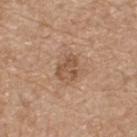A male subject, approximately 70 years of age. The lesion-visualizer software estimated a footprint of about 6.5 mm², a shape eccentricity near 0.55, and a symmetry-axis asymmetry near 0.3. And it measured an average lesion color of about L≈53 a*≈19 b*≈31 (CIELAB), about 9 CIELAB-L* units darker than the surrounding skin, and a lesion-to-skin contrast of about 6.5 (normalized; higher = more distinct). It also reported a border-irregularity index near 3/10, a color-variation rating of about 3.5/10, and radial color variation of about 1. The software also gave a nevus-likeness score of about 0/100 and a lesion-detection confidence of about 100/100. On the upper back. About 3.5 mm across. A 15 mm close-up extracted from a 3D total-body photography capture.